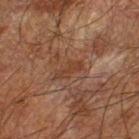| key | value |
|---|---|
| workup | catalogued during a skin exam; not biopsied |
| automated lesion analysis | a lesion area of about 3.5 mm², an outline eccentricity of about 0.9 (0 = round, 1 = elongated), and a shape-asymmetry score of about 0.4 (0 = symmetric); an average lesion color of about L≈32 a*≈18 b*≈26 (CIELAB), about 5 CIELAB-L* units darker than the surrounding skin, and a normalized border contrast of about 5.5; a border-irregularity index near 5/10 and internal color variation of about 0 on a 0–10 scale; a classifier nevus-likeness of about 0/100 and a detector confidence of about 70 out of 100 that the crop contains a lesion |
| size | ≈3.5 mm |
| imaging modality | 15 mm crop, total-body photography |
| location | the left forearm |
| patient | male, in their mid-60s |